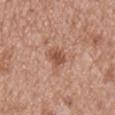The lesion was photographed on a routine skin check and not biopsied; there is no pathology result.
Approximately 2.5 mm at its widest.
A 15 mm close-up tile from a total-body photography series done for melanoma screening.
The tile uses white-light illumination.
The patient is a male in their mid-60s.
On the mid back.